The lesion is on the left forearm. A female patient, approximately 80 years of age. A lesion tile, about 15 mm wide, cut from a 3D total-body photograph — 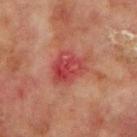<record>
<lighting>cross-polarized</lighting>
<lesion_size>
  <long_diameter_mm_approx>5.0</long_diameter_mm_approx>
</lesion_size>
<diagnosis>
  <histopathology>nodular basal cell carcinoma</histopathology>
  <malignancy>malignant</malignancy>
  <taxonomic_path>Malignant; Malignant adnexal epithelial proliferations - Follicular; Basal cell carcinoma; Basal cell carcinoma, Nodular</taxonomic_path>
</diagnosis>
</record>